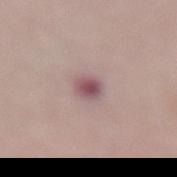Part of a total-body skin-imaging series; this lesion was reviewed on a skin check and was not flagged for biopsy. The patient is a female roughly 65 years of age. A region of skin cropped from a whole-body photographic capture, roughly 15 mm wide. On the lower back.